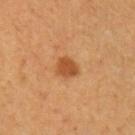biopsy status = no biopsy performed (imaged during a skin exam); location = the left upper arm; subject = female, aged approximately 45; tile lighting = cross-polarized illumination; image source = total-body-photography crop, ~15 mm field of view.